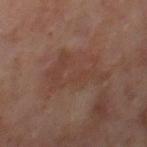The lesion was photographed on a routine skin check and not biopsied; there is no pathology result. Measured at roughly 6.5 mm in maximum diameter. Located on the right thigh. The subject is a female aged approximately 60. The tile uses cross-polarized illumination. A lesion tile, about 15 mm wide, cut from a 3D total-body photograph.